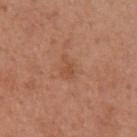Q: Was a biopsy performed?
A: no biopsy performed (imaged during a skin exam)
Q: Where on the body is the lesion?
A: the left upper arm
Q: How was this image acquired?
A: ~15 mm crop, total-body skin-cancer survey
Q: Patient demographics?
A: female, aged 28 to 32
Q: Automated lesion metrics?
A: a lesion area of about 4 mm², an eccentricity of roughly 0.8, and a shape-asymmetry score of about 0.4 (0 = symmetric); a border-irregularity index near 4/10, internal color variation of about 1.5 on a 0–10 scale, and a peripheral color-asymmetry measure near 0.5
Q: How was the tile lit?
A: white-light
Q: How large is the lesion?
A: ~3 mm (longest diameter)